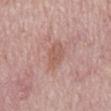{
  "biopsy_status": "not biopsied; imaged during a skin examination",
  "site": "mid back",
  "patient": {
    "sex": "male",
    "age_approx": 75
  },
  "lesion_size": {
    "long_diameter_mm_approx": 4.0
  },
  "lighting": "white-light",
  "image": {
    "source": "total-body photography crop",
    "field_of_view_mm": 15
  }
}